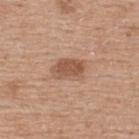Automated tile analysis of the lesion measured an area of roughly 8.5 mm², a shape eccentricity near 0.8, and a symmetry-axis asymmetry near 0.2. It also reported a lesion-to-skin contrast of about 7.5 (normalized; higher = more distinct). The software also gave a border-irregularity index near 2/10. The recorded lesion diameter is about 4 mm. A 15 mm close-up tile from a total-body photography series done for melanoma screening. Captured under white-light illumination. The lesion is on the upper back. A female subject, about 60 years old.